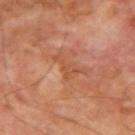Imaged during a routine full-body skin examination; the lesion was not biopsied and no histopathology is available. An algorithmic analysis of the crop reported a border-irregularity index near 8.5/10, internal color variation of about 0.5 on a 0–10 scale, and radial color variation of about 0. The analysis additionally found a classifier nevus-likeness of about 0/100 and a lesion-detection confidence of about 100/100. Captured under cross-polarized illumination. The lesion's longest dimension is about 3.5 mm. A male subject, roughly 70 years of age. Cropped from a total-body skin-imaging series; the visible field is about 15 mm. From the right upper arm.